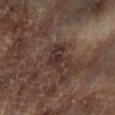No biopsy was performed on this lesion — it was imaged during a full skin examination and was not determined to be concerning. On the left lower leg. Captured under cross-polarized illumination. A 15 mm close-up extracted from a 3D total-body photography capture. The lesion-visualizer software estimated an automated nevus-likeness rating near 0 out of 100 and lesion-presence confidence of about 90/100. A male subject aged 63 to 67.